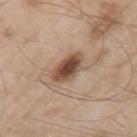notes = catalogued during a skin exam; not biopsied | body site = the left upper arm | patient = male, approximately 55 years of age | tile lighting = white-light illumination | acquisition = 15 mm crop, total-body photography | lesion diameter = ~4.5 mm (longest diameter).